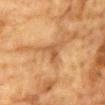Imaged during a routine full-body skin examination; the lesion was not biopsied and no histopathology is available. Approximately 3 mm at its widest. A male patient, aged around 85 or older. The lesion is located on the chest. Captured under cross-polarized illumination. Cropped from a total-body skin-imaging series; the visible field is about 15 mm.